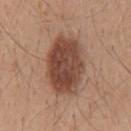Case summary:
• follow-up · imaged on a skin check; not biopsied
• site · the mid back
• acquisition · ~15 mm tile from a whole-body skin photo
• subject · male, aged around 40